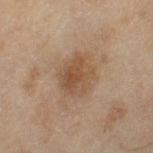The lesion is on the right thigh. Automated tile analysis of the lesion measured a lesion area of about 13 mm² and an eccentricity of roughly 0.55. It also reported a lesion color around L≈46 a*≈16 b*≈29 in CIELAB, roughly 8 lightness units darker than nearby skin, and a lesion-to-skin contrast of about 6.5 (normalized; higher = more distinct). The software also gave a lesion-detection confidence of about 100/100. Cropped from a total-body skin-imaging series; the visible field is about 15 mm. Captured under cross-polarized illumination. A female patient about 60 years old.